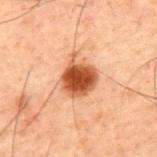Clinical impression:
Imaged during a routine full-body skin examination; the lesion was not biopsied and no histopathology is available.